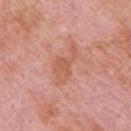Part of a total-body skin-imaging series; this lesion was reviewed on a skin check and was not flagged for biopsy. A male subject in their mid- to late 60s. From the upper back. A region of skin cropped from a whole-body photographic capture, roughly 15 mm wide.